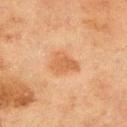No biopsy was performed on this lesion — it was imaged during a full skin examination and was not determined to be concerning. Captured under cross-polarized illumination. The lesion-visualizer software estimated a mean CIELAB color near L≈50 a*≈21 b*≈34, a lesion–skin lightness drop of about 8, and a normalized lesion–skin contrast near 6.5. And it measured a classifier nevus-likeness of about 65/100. A 15 mm close-up extracted from a 3D total-body photography capture. The subject is a male aged 68 to 72. Longest diameter approximately 3.5 mm. The lesion is on the upper back.